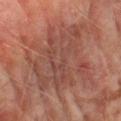workup: total-body-photography surveillance lesion; no biopsy
anatomic site: the left thigh
image source: ~15 mm tile from a whole-body skin photo
lesion diameter: ~9.5 mm (longest diameter)
subject: male, aged around 75
automated lesion analysis: a shape eccentricity near 0.35 and a shape-asymmetry score of about 0.4 (0 = symmetric); roughly 5 lightness units darker than nearby skin
lighting: cross-polarized